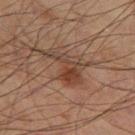{
  "biopsy_status": "not biopsied; imaged during a skin examination",
  "lesion_size": {
    "long_diameter_mm_approx": 6.0
  },
  "image": {
    "source": "total-body photography crop",
    "field_of_view_mm": 15
  },
  "patient": {
    "sex": "male",
    "age_approx": 70
  },
  "lighting": "cross-polarized",
  "site": "left thigh"
}